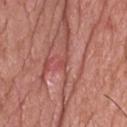| feature | finding |
|---|---|
| biopsy status | imaged on a skin check; not biopsied |
| site | the head or neck |
| diameter | ~1 mm (longest diameter) |
| illumination | white-light |
| imaging modality | 15 mm crop, total-body photography |
| image-analysis metrics | a lesion color around L≈49 a*≈32 b*≈26 in CIELAB and a normalized border contrast of about 5; border irregularity of about 2 on a 0–10 scale and a peripheral color-asymmetry measure near 0; a nevus-likeness score of about 0/100 and lesion-presence confidence of about 5/100 |
| patient | male, aged around 60 |